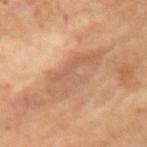Recorded during total-body skin imaging; not selected for excision or biopsy. Approximately 7.5 mm at its widest. Automated tile analysis of the lesion measured a footprint of about 15 mm², an outline eccentricity of about 0.9 (0 = round, 1 = elongated), and a symmetry-axis asymmetry near 0.45. It also reported an average lesion color of about L≈57 a*≈20 b*≈32 (CIELAB), a lesion–skin lightness drop of about 7, and a normalized lesion–skin contrast near 5. This image is a 15 mm lesion crop taken from a total-body photograph. Captured under cross-polarized illumination. A female subject aged approximately 70. The lesion is located on the left upper arm.